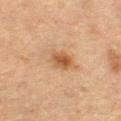Case summary:
- biopsy status — catalogued during a skin exam; not biopsied
- image — ~15 mm tile from a whole-body skin photo
- location — the right thigh
- patient — female, aged 53–57
- illumination — cross-polarized illumination